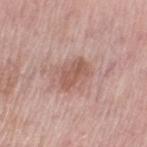Q: Patient demographics?
A: female, in their 60s
Q: What is the imaging modality?
A: total-body-photography crop, ~15 mm field of view
Q: What is the anatomic site?
A: the left upper arm